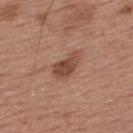{
  "biopsy_status": "not biopsied; imaged during a skin examination",
  "patient": {
    "sex": "male",
    "age_approx": 55
  },
  "image": {
    "source": "total-body photography crop",
    "field_of_view_mm": 15
  },
  "site": "upper back",
  "lighting": "white-light",
  "lesion_size": {
    "long_diameter_mm_approx": 3.5
  }
}